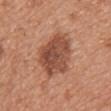Context: On the upper back. A region of skin cropped from a whole-body photographic capture, roughly 15 mm wide. Approximately 6 mm at its widest. Captured under white-light illumination. A female patient aged 33–37.